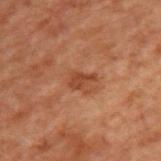Captured during whole-body skin photography for melanoma surveillance; the lesion was not biopsied. A male subject, aged 68 to 72. From the upper back. The lesion's longest dimension is about 2.5 mm. A 15 mm close-up extracted from a 3D total-body photography capture. Automated image analysis of the tile measured a mean CIELAB color near L≈33 a*≈21 b*≈28 and a normalized lesion–skin contrast near 7.5. The analysis additionally found a classifier nevus-likeness of about 25/100 and lesion-presence confidence of about 100/100.